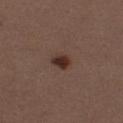Part of a total-body skin-imaging series; this lesion was reviewed on a skin check and was not flagged for biopsy. The lesion is located on the leg. The subject is a female aged around 40. A 15 mm close-up extracted from a 3D total-body photography capture.